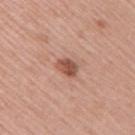{"biopsy_status": "not biopsied; imaged during a skin examination", "lighting": "white-light", "patient": {"sex": "male", "age_approx": 55}, "lesion_size": {"long_diameter_mm_approx": 2.5}, "site": "right upper arm", "image": {"source": "total-body photography crop", "field_of_view_mm": 15}, "automated_metrics": {"cielab_L": 52, "cielab_a": 24, "cielab_b": 29, "vs_skin_darker_L": 13.0, "vs_skin_contrast_norm": 9.0, "border_irregularity_0_10": 2.0, "color_variation_0_10": 3.5, "peripheral_color_asymmetry": 1.0, "nevus_likeness_0_100": 80}}